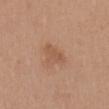Context:
Measured at roughly 3.5 mm in maximum diameter. Automated tile analysis of the lesion measured border irregularity of about 3.5 on a 0–10 scale, internal color variation of about 1.5 on a 0–10 scale, and radial color variation of about 0.5. The software also gave an automated nevus-likeness rating near 10 out of 100. The subject is a female aged 53 to 57. This is a white-light tile. The lesion is on the mid back. A lesion tile, about 15 mm wide, cut from a 3D total-body photograph.